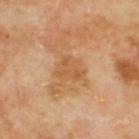Background:
Cropped from a whole-body photographic skin survey; the tile spans about 15 mm. The total-body-photography lesion software estimated an average lesion color of about L≈55 a*≈21 b*≈39 (CIELAB), a lesion–skin lightness drop of about 6, and a lesion-to-skin contrast of about 5 (normalized; higher = more distinct). The software also gave internal color variation of about 2.5 on a 0–10 scale and a peripheral color-asymmetry measure near 0.5. The lesion is on the back. A male subject, aged 68 to 72. This is a cross-polarized tile.